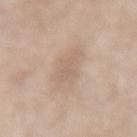Case summary:
– follow-up · imaged on a skin check; not biopsied